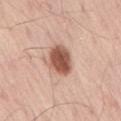biopsy_status: not biopsied; imaged during a skin examination